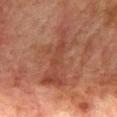Clinical impression: Captured during whole-body skin photography for melanoma surveillance; the lesion was not biopsied. Acquisition and patient details: A lesion tile, about 15 mm wide, cut from a 3D total-body photograph. Approximately 8.5 mm at its widest. The subject is a male aged approximately 75. Captured under cross-polarized illumination. The lesion is on the mid back.